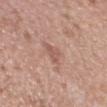Background: The lesion is on the right forearm. A close-up tile cropped from a whole-body skin photograph, about 15 mm across. Approximately 2.5 mm at its widest. A female subject aged around 40.